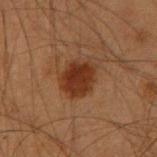Notes:
* biopsy status · catalogued during a skin exam; not biopsied
* body site · the right forearm
* subject · male, in their mid- to late 50s
* image source · total-body-photography crop, ~15 mm field of view